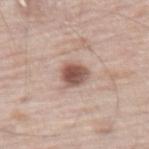Q: Was this lesion biopsied?
A: total-body-photography surveillance lesion; no biopsy